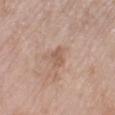Impression: Part of a total-body skin-imaging series; this lesion was reviewed on a skin check and was not flagged for biopsy.